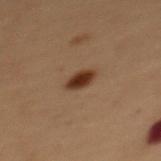biopsy status: catalogued during a skin exam; not biopsied | image: ~15 mm tile from a whole-body skin photo | location: the back | size: about 2.5 mm | lighting: cross-polarized | subject: female, aged 48–52.